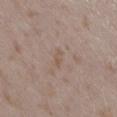Q: Was this lesion biopsied?
A: total-body-photography surveillance lesion; no biopsy
Q: What is the imaging modality?
A: ~15 mm crop, total-body skin-cancer survey
Q: How was the tile lit?
A: white-light
Q: Lesion location?
A: the lower back
Q: Patient demographics?
A: female, roughly 30 years of age
Q: Lesion size?
A: about 2.5 mm